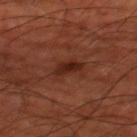The lesion was photographed on a routine skin check and not biopsied; there is no pathology result. The lesion is on the right thigh. A roughly 15 mm field-of-view crop from a total-body skin photograph. Imaged with cross-polarized lighting. The subject is roughly 65 years of age.